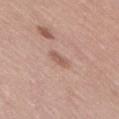No biopsy was performed on this lesion — it was imaged during a full skin examination and was not determined to be concerning. The lesion's longest dimension is about 3.5 mm. A lesion tile, about 15 mm wide, cut from a 3D total-body photograph. The lesion-visualizer software estimated a lesion area of about 5.5 mm², a shape eccentricity near 0.85, and a symmetry-axis asymmetry near 0.25. And it measured a border-irregularity rating of about 2.5/10, a within-lesion color-variation index near 2.5/10, and a peripheral color-asymmetry measure near 1. The software also gave an automated nevus-likeness rating near 0 out of 100 and a detector confidence of about 100 out of 100 that the crop contains a lesion. On the right thigh. The tile uses white-light illumination. A female patient, aged 38 to 42.